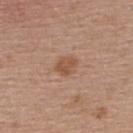A lesion tile, about 15 mm wide, cut from a 3D total-body photograph. The patient is a female aged approximately 40. Automated image analysis of the tile measured a footprint of about 4.5 mm², an outline eccentricity of about 0.6 (0 = round, 1 = elongated), and a shape-asymmetry score of about 0.25 (0 = symmetric). And it measured roughly 9 lightness units darker than nearby skin and a lesion-to-skin contrast of about 6.5 (normalized; higher = more distinct). And it measured a border-irregularity rating of about 2/10, a within-lesion color-variation index near 2/10, and a peripheral color-asymmetry measure near 0.5. On the upper back. The tile uses white-light illumination.